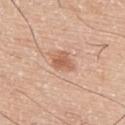Imaged during a routine full-body skin examination; the lesion was not biopsied and no histopathology is available. A male subject, aged around 75. Located on the upper back. The tile uses white-light illumination. An algorithmic analysis of the crop reported a lesion area of about 5.5 mm² and a shape-asymmetry score of about 0.3 (0 = symmetric). The analysis additionally found border irregularity of about 2.5 on a 0–10 scale and a peripheral color-asymmetry measure near 1. A 15 mm crop from a total-body photograph taken for skin-cancer surveillance.